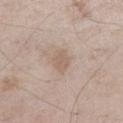notes: imaged on a skin check; not biopsied.